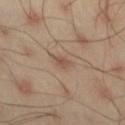– follow-up: catalogued during a skin exam; not biopsied
– illumination: cross-polarized illumination
– subject: male, roughly 45 years of age
– TBP lesion metrics: a mean CIELAB color near L≈50 a*≈17 b*≈26; an automated nevus-likeness rating near 5 out of 100 and a lesion-detection confidence of about 100/100
– image source: 15 mm crop, total-body photography
– lesion diameter: ~2.5 mm (longest diameter)
– site: the right thigh